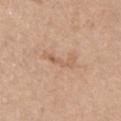Q: Was a biopsy performed?
A: imaged on a skin check; not biopsied
Q: Patient demographics?
A: male, approximately 70 years of age
Q: Automated lesion metrics?
A: a footprint of about 4 mm², an eccentricity of roughly 0.95, and two-axis asymmetry of about 0.55; an average lesion color of about L≈60 a*≈19 b*≈33 (CIELAB), roughly 7 lightness units darker than nearby skin, and a lesion-to-skin contrast of about 5 (normalized; higher = more distinct)
Q: Lesion size?
A: ~4 mm (longest diameter)
Q: Where on the body is the lesion?
A: the chest
Q: Illumination type?
A: white-light illumination
Q: How was this image acquired?
A: total-body-photography crop, ~15 mm field of view